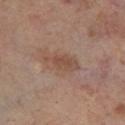notes = catalogued during a skin exam; not biopsied | size = about 4.5 mm | acquisition = total-body-photography crop, ~15 mm field of view | subject = male, approximately 70 years of age | anatomic site = the right lower leg | tile lighting = cross-polarized illumination.